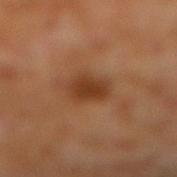* notes: total-body-photography surveillance lesion; no biopsy
* image: ~15 mm crop, total-body skin-cancer survey
* patient: male, aged around 60
* site: the right lower leg